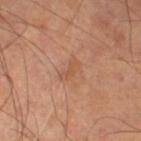  patient:
    sex: male
    age_approx: 70
  site: left lower leg
  image:
    source: total-body photography crop
    field_of_view_mm: 15